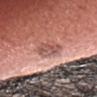biopsy status = no biopsy performed (imaged during a skin exam)
location = the head or neck
image = ~15 mm tile from a whole-body skin photo
patient = male, aged 38–42
size = about 3 mm
illumination = white-light